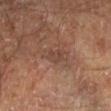Q: Who is the patient?
A: male, aged approximately 60
Q: Where on the body is the lesion?
A: the left lower leg
Q: What kind of image is this?
A: ~15 mm crop, total-body skin-cancer survey
Q: What is the lesion's diameter?
A: ~2.5 mm (longest diameter)
Q: Illumination type?
A: cross-polarized